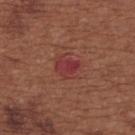| key | value |
|---|---|
| follow-up | catalogued during a skin exam; not biopsied |
| subject | female, aged 63 to 67 |
| tile lighting | white-light illumination |
| lesion size | about 3 mm |
| acquisition | ~15 mm crop, total-body skin-cancer survey |
| anatomic site | the upper back |